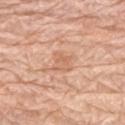Clinical impression:
Recorded during total-body skin imaging; not selected for excision or biopsy.
Context:
This image is a 15 mm lesion crop taken from a total-body photograph. Measured at roughly 2.5 mm in maximum diameter. This is a white-light tile. Located on the upper back. An algorithmic analysis of the crop reported border irregularity of about 3.5 on a 0–10 scale, internal color variation of about 2.5 on a 0–10 scale, and radial color variation of about 1. And it measured lesion-presence confidence of about 100/100. The subject is a female aged around 65.